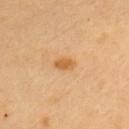* workup · no biopsy performed (imaged during a skin exam)
* diameter · ≈2.5 mm
* imaging modality · ~15 mm crop, total-body skin-cancer survey
* patient · female, aged around 60
* body site · the left upper arm
* automated metrics · internal color variation of about 2 on a 0–10 scale and a peripheral color-asymmetry measure near 1; a nevus-likeness score of about 80/100 and a detector confidence of about 100 out of 100 that the crop contains a lesion
* tile lighting · cross-polarized illumination